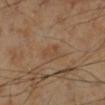notes=catalogued during a skin exam; not biopsied
patient=male, in their 70s
lesion diameter=~3 mm (longest diameter)
illumination=cross-polarized
image=~15 mm crop, total-body skin-cancer survey
body site=the right lower leg